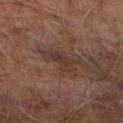Part of a total-body skin-imaging series; this lesion was reviewed on a skin check and was not flagged for biopsy.
On the right lower leg.
An algorithmic analysis of the crop reported a nevus-likeness score of about 0/100 and a lesion-detection confidence of about 55/100.
Cropped from a whole-body photographic skin survey; the tile spans about 15 mm.
Approximately 4 mm at its widest.
A male patient, in their mid-70s.